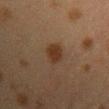Findings:
– follow-up: no biopsy performed (imaged during a skin exam)
– diameter: about 3 mm
– imaging modality: 15 mm crop, total-body photography
– automated metrics: an average lesion color of about L≈28 a*≈15 b*≈25 (CIELAB), roughly 7 lightness units darker than nearby skin, and a normalized border contrast of about 8.5; a border-irregularity index near 1.5/10 and internal color variation of about 2 on a 0–10 scale; a nevus-likeness score of about 95/100 and a detector confidence of about 100 out of 100 that the crop contains a lesion
– subject: female, aged 38 to 42
– tile lighting: cross-polarized
– body site: the front of the torso